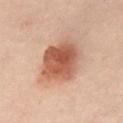Clinical impression:
The lesion was tiled from a total-body skin photograph and was not biopsied.
Image and clinical context:
A 15 mm close-up extracted from a 3D total-body photography capture. The patient is a male in their 50s. The recorded lesion diameter is about 5.5 mm. The lesion is located on the abdomen. Captured under cross-polarized illumination.